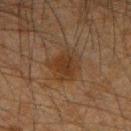{"biopsy_status": "not biopsied; imaged during a skin examination", "site": "right forearm", "patient": {"sex": "male", "age_approx": 35}, "lighting": "cross-polarized", "image": {"source": "total-body photography crop", "field_of_view_mm": 15}}